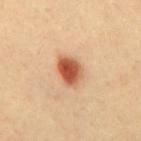Clinical summary:
A 15 mm crop from a total-body photograph taken for skin-cancer surveillance. The tile uses cross-polarized illumination. A male patient, in their 40s. The lesion's longest dimension is about 4 mm. Located on the mid back.